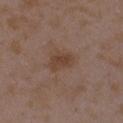Impression:
Imaged during a routine full-body skin examination; the lesion was not biopsied and no histopathology is available.
Acquisition and patient details:
Cropped from a total-body skin-imaging series; the visible field is about 15 mm. On the left upper arm. A female patient, about 35 years old.